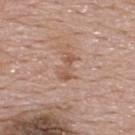<case>
<biopsy_status>not biopsied; imaged during a skin examination</biopsy_status>
<site>upper back</site>
<patient>
  <sex>male</sex>
  <age_approx>55</age_approx>
</patient>
<automated_metrics>
  <border_irregularity_0_10>5.5</border_irregularity_0_10>
  <color_variation_0_10>0.0</color_variation_0_10>
  <nevus_likeness_0_100>0</nevus_likeness_0_100>
</automated_metrics>
<image>
  <source>total-body photography crop</source>
  <field_of_view_mm>15</field_of_view_mm>
</image>
<lesion_size>
  <long_diameter_mm_approx>3.0</long_diameter_mm_approx>
</lesion_size>
<lighting>white-light</lighting>
</case>